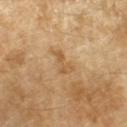The lesion was photographed on a routine skin check and not biopsied; there is no pathology result.
On the left forearm.
The recorded lesion diameter is about 3.5 mm.
Cropped from a whole-body photographic skin survey; the tile spans about 15 mm.
A female subject, about 70 years old.
An algorithmic analysis of the crop reported a border-irregularity rating of about 6.5/10, a color-variation rating of about 2/10, and peripheral color asymmetry of about 0.5.
The tile uses cross-polarized illumination.